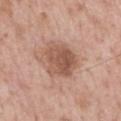notes: total-body-photography surveillance lesion; no biopsy
lighting: white-light illumination
diameter: about 4 mm
imaging modality: total-body-photography crop, ~15 mm field of view
image-analysis metrics: an area of roughly 13 mm² and an outline eccentricity of about 0.4 (0 = round, 1 = elongated); a mean CIELAB color near L≈54 a*≈21 b*≈29, roughly 11 lightness units darker than nearby skin, and a lesion-to-skin contrast of about 7.5 (normalized; higher = more distinct)
location: the mid back
subject: male, aged around 65